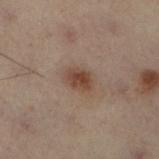<tbp_lesion>
<biopsy_status>not biopsied; imaged during a skin examination</biopsy_status>
<patient>
  <sex>female</sex>
  <age_approx>55</age_approx>
</patient>
<lighting>cross-polarized</lighting>
<image>
  <source>total-body photography crop</source>
  <field_of_view_mm>15</field_of_view_mm>
</image>
<site>left leg</site>
<lesion_size>
  <long_diameter_mm_approx>3.0</long_diameter_mm_approx>
</lesion_size>
<automated_metrics>
  <cielab_L>36</cielab_L>
  <cielab_a>15</cielab_a>
  <cielab_b>22</cielab_b>
  <vs_skin_darker_L>9.0</vs_skin_darker_L>
  <vs_skin_contrast_norm>8.0</vs_skin_contrast_norm>
</automated_metrics>
</tbp_lesion>